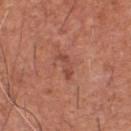Captured during whole-body skin photography for melanoma surveillance; the lesion was not biopsied. A 15 mm close-up extracted from a 3D total-body photography capture. The patient is a male aged approximately 65. Measured at roughly 3.5 mm in maximum diameter. Automated tile analysis of the lesion measured an area of roughly 3.5 mm² and an outline eccentricity of about 0.9 (0 = round, 1 = elongated). And it measured an average lesion color of about L≈48 a*≈26 b*≈29 (CIELAB), roughly 8 lightness units darker than nearby skin, and a normalized lesion–skin contrast near 6. The software also gave a border-irregularity index near 5.5/10 and a color-variation rating of about 0/10. The software also gave a detector confidence of about 100 out of 100 that the crop contains a lesion. This is a white-light tile. Located on the chest.